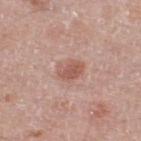workup=catalogued during a skin exam; not biopsied | image source=~15 mm tile from a whole-body skin photo | anatomic site=the right thigh | patient=male, approximately 80 years of age | lighting=white-light | lesion diameter=about 3 mm.